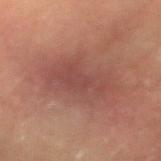Recorded during total-body skin imaging; not selected for excision or biopsy.
On the left forearm.
The patient is a male in their 60s.
Cropped from a total-body skin-imaging series; the visible field is about 15 mm.
Captured under cross-polarized illumination.